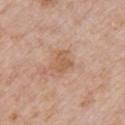follow-up: catalogued during a skin exam; not biopsied | size: ≈3 mm | automated lesion analysis: a footprint of about 4.5 mm², an outline eccentricity of about 0.55 (0 = round, 1 = elongated), and a symmetry-axis asymmetry near 0.4; a lesion color around L≈58 a*≈20 b*≈33 in CIELAB, a lesion–skin lightness drop of about 8, and a normalized lesion–skin contrast near 6; a border-irregularity index near 4/10, a color-variation rating of about 1.5/10, and a peripheral color-asymmetry measure near 0.5; a classifier nevus-likeness of about 0/100 and lesion-presence confidence of about 100/100 | lighting: white-light illumination | subject: female, in their 70s | anatomic site: the chest | image source: 15 mm crop, total-body photography.